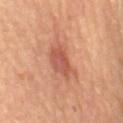Imaged during a routine full-body skin examination; the lesion was not biopsied and no histopathology is available.
The tile uses cross-polarized illumination.
A female patient aged 68–72.
From the back.
A 15 mm crop from a total-body photograph taken for skin-cancer surveillance.